A region of skin cropped from a whole-body photographic capture, roughly 15 mm wide · a female patient, aged around 40 · on the left upper arm:
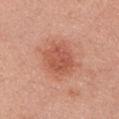Approximately 4 mm at its widest. Automated tile analysis of the lesion measured a footprint of about 11 mm², an outline eccentricity of about 0.6 (0 = round, 1 = elongated), and two-axis asymmetry of about 0.15. The analysis additionally found an average lesion color of about L≈55 a*≈29 b*≈32 (CIELAB). The analysis additionally found a border-irregularity rating of about 2/10, a within-lesion color-variation index near 3/10, and peripheral color asymmetry of about 1. The analysis additionally found an automated nevus-likeness rating near 60 out of 100 and a detector confidence of about 100 out of 100 that the crop contains a lesion. Captured under white-light illumination. Histopathology of the biopsied lesion showed a compound melanocytic nevus.